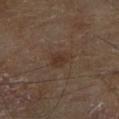Q: What lighting was used for the tile?
A: cross-polarized illumination
Q: How large is the lesion?
A: about 2.5 mm
Q: What is the imaging modality?
A: 15 mm crop, total-body photography
Q: Automated lesion metrics?
A: a footprint of about 4.5 mm², a shape eccentricity near 0.6, and a shape-asymmetry score of about 0.25 (0 = symmetric); a border-irregularity rating of about 2.5/10, a color-variation rating of about 1.5/10, and peripheral color asymmetry of about 0.5; an automated nevus-likeness rating near 10 out of 100 and a lesion-detection confidence of about 100/100
Q: Where on the body is the lesion?
A: the right lower leg
Q: Patient demographics?
A: male, in their 70s